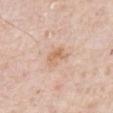The lesion was photographed on a routine skin check and not biopsied; there is no pathology result.
The lesion's longest dimension is about 3 mm.
Located on the abdomen.
Cropped from a total-body skin-imaging series; the visible field is about 15 mm.
This is a white-light tile.
A male subject, roughly 80 years of age.